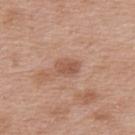<lesion>
  <biopsy_status>not biopsied; imaged during a skin examination</biopsy_status>
  <site>upper back</site>
  <image>
    <source>total-body photography crop</source>
    <field_of_view_mm>15</field_of_view_mm>
  </image>
  <patient>
    <sex>female</sex>
    <age_approx>40</age_approx>
  </patient>
</lesion>